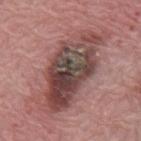Impression:
Captured during whole-body skin photography for melanoma surveillance; the lesion was not biopsied.
Image and clinical context:
A 15 mm close-up tile from a total-body photography series done for melanoma screening. Approximately 11 mm at its widest. The lesion is on the mid back. The tile uses white-light illumination. A male patient, aged 68–72.